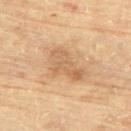Captured during whole-body skin photography for melanoma surveillance; the lesion was not biopsied.
A female subject roughly 80 years of age.
From the upper back.
A lesion tile, about 15 mm wide, cut from a 3D total-body photograph.